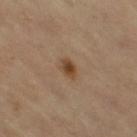Q: Was a biopsy performed?
A: catalogued during a skin exam; not biopsied
Q: Where on the body is the lesion?
A: the right thigh
Q: Patient demographics?
A: female, about 70 years old
Q: Illumination type?
A: cross-polarized
Q: How large is the lesion?
A: ~3 mm (longest diameter)
Q: What is the imaging modality?
A: 15 mm crop, total-body photography
Q: What did automated image analysis measure?
A: a lesion area of about 4 mm², a shape eccentricity near 0.85, and two-axis asymmetry of about 0.25; a border-irregularity index near 2.5/10 and internal color variation of about 4 on a 0–10 scale; a classifier nevus-likeness of about 95/100